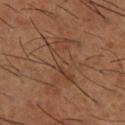{"biopsy_status": "not biopsied; imaged during a skin examination", "lighting": "cross-polarized", "patient": {"sex": "male", "age_approx": 65}, "lesion_size": {"long_diameter_mm_approx": 7.0}, "site": "right lower leg", "image": {"source": "total-body photography crop", "field_of_view_mm": 15}, "automated_metrics": {"area_mm2_approx": 16.0, "eccentricity": 0.9, "cielab_L": 41, "cielab_a": 20, "cielab_b": 29, "vs_skin_darker_L": 5.0, "vs_skin_contrast_norm": 4.5, "border_irregularity_0_10": 8.0, "color_variation_0_10": 5.5, "peripheral_color_asymmetry": 2.0, "nevus_likeness_0_100": 0, "lesion_detection_confidence_0_100": 95}}